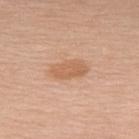Clinical summary: Longest diameter approximately 4 mm. This is a white-light tile. The lesion is located on the upper back. A roughly 15 mm field-of-view crop from a total-body skin photograph. Automated image analysis of the tile measured a footprint of about 6.5 mm², a shape eccentricity near 0.85, and two-axis asymmetry of about 0.2. And it measured peripheral color asymmetry of about 0.5. A female patient, about 55 years old.